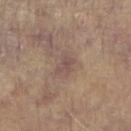{"biopsy_status": "not biopsied; imaged during a skin examination", "site": "right forearm", "image": {"source": "total-body photography crop", "field_of_view_mm": 15}, "patient": {"sex": "male", "age_approx": 60}, "lesion_size": {"long_diameter_mm_approx": 2.5}}